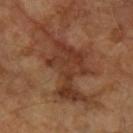Captured during whole-body skin photography for melanoma surveillance; the lesion was not biopsied.
A female subject, aged around 70.
The total-body-photography lesion software estimated two-axis asymmetry of about 0.55. And it measured border irregularity of about 9 on a 0–10 scale, internal color variation of about 3.5 on a 0–10 scale, and radial color variation of about 1. The analysis additionally found a classifier nevus-likeness of about 0/100 and lesion-presence confidence of about 85/100.
A close-up tile cropped from a whole-body skin photograph, about 15 mm across.
From the arm.
Imaged with cross-polarized lighting.
Measured at roughly 10.5 mm in maximum diameter.